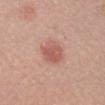Case summary:
- workup · catalogued during a skin exam; not biopsied
- patient · female, aged 53 to 57
- lighting · white-light illumination
- imaging modality · total-body-photography crop, ~15 mm field of view
- site · the head or neck